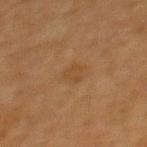This lesion was catalogued during total-body skin photography and was not selected for biopsy. Approximately 2.5 mm at its widest. Imaged with cross-polarized lighting. A female subject, about 55 years old. The lesion is located on the upper back. A close-up tile cropped from a whole-body skin photograph, about 15 mm across.